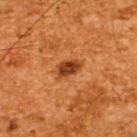workup = imaged on a skin check; not biopsied | size = about 3 mm | patient = male, aged 63 to 67 | illumination = cross-polarized illumination | image source = ~15 mm crop, total-body skin-cancer survey | location = the upper back.